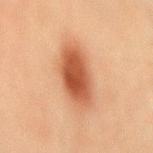{
  "site": "abdomen",
  "image": {
    "source": "total-body photography crop",
    "field_of_view_mm": 15
  },
  "lighting": "cross-polarized",
  "automated_metrics": {
    "border_irregularity_0_10": 1.5,
    "color_variation_0_10": 4.5,
    "peripheral_color_asymmetry": 1.5
  },
  "patient": {
    "sex": "male",
    "age_approx": 70
  }
}